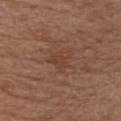workup: catalogued during a skin exam; not biopsied
automated metrics: a lesion color around L≈41 a*≈20 b*≈28 in CIELAB, roughly 5 lightness units darker than nearby skin, and a normalized border contrast of about 4.5; a border-irregularity index near 4.5/10 and a peripheral color-asymmetry measure near 1
subject: female, aged 38 to 42
size: ≈3.5 mm
tile lighting: white-light illumination
location: the chest
acquisition: 15 mm crop, total-body photography